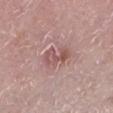Part of a total-body skin-imaging series; this lesion was reviewed on a skin check and was not flagged for biopsy.
A male subject, aged around 50.
The tile uses white-light illumination.
On the left lower leg.
The lesion's longest dimension is about 3.5 mm.
A 15 mm close-up extracted from a 3D total-body photography capture.
The lesion-visualizer software estimated a border-irregularity rating of about 5/10, internal color variation of about 3.5 on a 0–10 scale, and radial color variation of about 1. And it measured a nevus-likeness score of about 0/100 and a lesion-detection confidence of about 100/100.